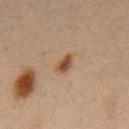Recorded during total-body skin imaging; not selected for excision or biopsy. On the mid back. The tile uses cross-polarized illumination. The subject is a male aged approximately 55. The lesion-visualizer software estimated a footprint of about 3.5 mm², an eccentricity of roughly 0.8, and a shape-asymmetry score of about 0.25 (0 = symmetric). It also reported a mean CIELAB color near L≈49 a*≈20 b*≈34 and a lesion–skin lightness drop of about 11. Cropped from a total-body skin-imaging series; the visible field is about 15 mm.